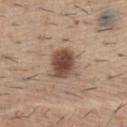biopsy status = imaged on a skin check; not biopsied.